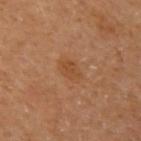follow-up: catalogued during a skin exam; not biopsied | location: the arm | tile lighting: cross-polarized | subject: female, aged around 60 | image source: ~15 mm tile from a whole-body skin photo.